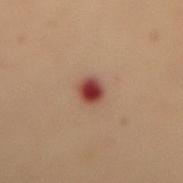Context: The lesion-visualizer software estimated a within-lesion color-variation index near 4.5/10 and a peripheral color-asymmetry measure near 1. It also reported an automated nevus-likeness rating near 0 out of 100 and a lesion-detection confidence of about 100/100. The lesion is located on the mid back. A 15 mm close-up tile from a total-body photography series done for melanoma screening. The tile uses cross-polarized illumination. The subject is a female aged 48–52.